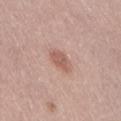notes: total-body-photography surveillance lesion; no biopsy
diameter: about 3 mm
subject: female, aged around 40
location: the lower back
tile lighting: white-light illumination
acquisition: 15 mm crop, total-body photography